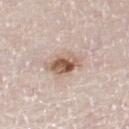Assessment:
The lesion was tiled from a total-body skin photograph and was not biopsied.